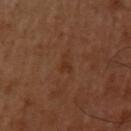Recorded during total-body skin imaging; not selected for excision or biopsy.
A male subject, aged approximately 50.
Located on the arm.
Measured at roughly 2.5 mm in maximum diameter.
The total-body-photography lesion software estimated a shape eccentricity near 0.9 and a shape-asymmetry score of about 0.6 (0 = symmetric).
The tile uses cross-polarized illumination.
A 15 mm close-up tile from a total-body photography series done for melanoma screening.